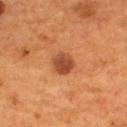notes=no biopsy performed (imaged during a skin exam) | lighting=cross-polarized | location=the upper back | subject=male, aged 53–57 | lesion diameter=~3 mm (longest diameter) | acquisition=15 mm crop, total-body photography.